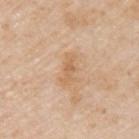Q: Is there a histopathology result?
A: no biopsy performed (imaged during a skin exam)
Q: Automated lesion metrics?
A: a lesion area of about 3.5 mm² and an outline eccentricity of about 0.9 (0 = round, 1 = elongated); border irregularity of about 4 on a 0–10 scale, a color-variation rating of about 0.5/10, and a peripheral color-asymmetry measure near 0
Q: Illumination type?
A: white-light
Q: Where on the body is the lesion?
A: the left upper arm
Q: What are the patient's age and sex?
A: male, aged approximately 75
Q: What is the imaging modality?
A: ~15 mm tile from a whole-body skin photo
Q: What is the lesion's diameter?
A: ~3 mm (longest diameter)